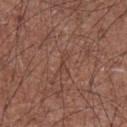The lesion was tiled from a total-body skin photograph and was not biopsied.
The total-body-photography lesion software estimated a lesion area of about 2 mm², an eccentricity of roughly 0.95, and a symmetry-axis asymmetry near 0.4. It also reported an average lesion color of about L≈42 a*≈20 b*≈25 (CIELAB), roughly 5 lightness units darker than nearby skin, and a normalized border contrast of about 4.5. The analysis additionally found a border-irregularity rating of about 4.5/10 and peripheral color asymmetry of about 0. And it measured a classifier nevus-likeness of about 0/100 and lesion-presence confidence of about 70/100.
A 15 mm crop from a total-body photograph taken for skin-cancer surveillance.
Longest diameter approximately 2.5 mm.
From the chest.
A male subject, aged 73 to 77.
The tile uses white-light illumination.